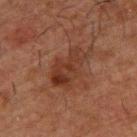Findings:
- anatomic site — the upper back
- subject — male, approximately 50 years of age
- lesion size — ≈5.5 mm
- image source — ~15 mm crop, total-body skin-cancer survey
- automated lesion analysis — a lesion area of about 12 mm² and a shape eccentricity near 0.85; a border-irregularity rating of about 4.5/10, a within-lesion color-variation index near 4.5/10, and a peripheral color-asymmetry measure near 1.5